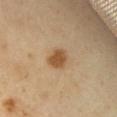biopsy status: no biopsy performed (imaged during a skin exam) | illumination: cross-polarized | subject: female, approximately 60 years of age | image: ~15 mm crop, total-body skin-cancer survey | body site: the chest | size: ~2.5 mm (longest diameter).